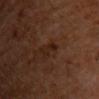Image and clinical context: The lesion is located on the upper back. This is a cross-polarized tile. Longest diameter approximately 2.5 mm. A lesion tile, about 15 mm wide, cut from a 3D total-body photograph. The subject is a male in their 60s.